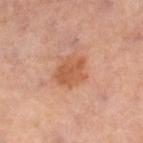Acquisition and patient details:
An algorithmic analysis of the crop reported a lesion area of about 9 mm², an outline eccentricity of about 0.6 (0 = round, 1 = elongated), and two-axis asymmetry of about 0.2. It also reported a border-irregularity rating of about 2.5/10, a within-lesion color-variation index near 2.5/10, and radial color variation of about 1. It also reported a nevus-likeness score of about 35/100 and lesion-presence confidence of about 100/100. On the leg. Cropped from a whole-body photographic skin survey; the tile spans about 15 mm. The lesion's longest dimension is about 4 mm. A female subject, approximately 50 years of age.